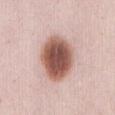Q: Is there a histopathology result?
A: no biopsy performed (imaged during a skin exam)
Q: What is the anatomic site?
A: the abdomen
Q: Patient demographics?
A: female, roughly 25 years of age
Q: Illumination type?
A: white-light illumination
Q: What is the lesion's diameter?
A: ~6 mm (longest diameter)
Q: Automated lesion metrics?
A: an area of roughly 21 mm² and a shape eccentricity near 0.65; an average lesion color of about L≈55 a*≈22 b*≈25 (CIELAB), roughly 20 lightness units darker than nearby skin, and a lesion-to-skin contrast of about 12.5 (normalized; higher = more distinct); a within-lesion color-variation index near 6/10 and radial color variation of about 1.5; a classifier nevus-likeness of about 100/100 and a detector confidence of about 100 out of 100 that the crop contains a lesion
Q: What kind of image is this?
A: ~15 mm crop, total-body skin-cancer survey